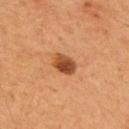A male patient, aged 53 to 57. A roughly 15 mm field-of-view crop from a total-body skin photograph. The lesion is on the upper back.